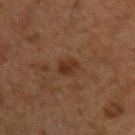Part of a total-body skin-imaging series; this lesion was reviewed on a skin check and was not flagged for biopsy.
About 2.5 mm across.
A male subject aged around 50.
A close-up tile cropped from a whole-body skin photograph, about 15 mm across.
The lesion is on the arm.